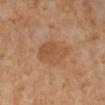This lesion was catalogued during total-body skin photography and was not selected for biopsy.
A female subject, about 50 years old.
A 15 mm close-up tile from a total-body photography series done for melanoma screening.
The recorded lesion diameter is about 4.5 mm.
The tile uses cross-polarized illumination.
Located on the right lower leg.